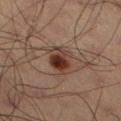workup: no biopsy performed (imaged during a skin exam) | lesion diameter: about 4.5 mm | subject: male, aged 58–62 | automated metrics: roughly 11 lightness units darker than nearby skin; border irregularity of about 2 on a 0–10 scale, a color-variation rating of about 9/10, and peripheral color asymmetry of about 3; a nevus-likeness score of about 95/100 and a detector confidence of about 100 out of 100 that the crop contains a lesion | image source: ~15 mm tile from a whole-body skin photo | tile lighting: cross-polarized illumination.